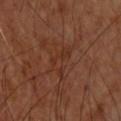Q: Was a biopsy performed?
A: total-body-photography surveillance lesion; no biopsy
Q: Lesion location?
A: the upper back
Q: Illumination type?
A: cross-polarized illumination
Q: What is the imaging modality?
A: total-body-photography crop, ~15 mm field of view
Q: Automated lesion metrics?
A: a border-irregularity rating of about 6/10, a within-lesion color-variation index near 3/10, and a peripheral color-asymmetry measure near 1
Q: Patient demographics?
A: male, aged 58–62